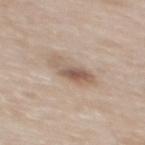* workup: imaged on a skin check; not biopsied
* automated metrics: an area of roughly 7 mm² and a shape eccentricity near 0.85
* size: about 4.5 mm
* anatomic site: the upper back
* image source: 15 mm crop, total-body photography
* subject: male, aged around 65
* illumination: white-light illumination